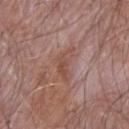Assessment: Captured during whole-body skin photography for melanoma surveillance; the lesion was not biopsied. Image and clinical context: Automated image analysis of the tile measured a lesion area of about 7 mm², an eccentricity of roughly 0.85, and a symmetry-axis asymmetry near 0.55. And it measured an automated nevus-likeness rating near 0 out of 100 and lesion-presence confidence of about 100/100. A male subject approximately 65 years of age. A 15 mm crop from a total-body photograph taken for skin-cancer surveillance. The lesion's longest dimension is about 4.5 mm. Located on the right forearm.